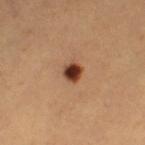Q: Is there a histopathology result?
A: catalogued during a skin exam; not biopsied
Q: Automated lesion metrics?
A: two-axis asymmetry of about 0.2; a mean CIELAB color near L≈40 a*≈24 b*≈32; a border-irregularity rating of about 1.5/10 and a color-variation rating of about 3.5/10; an automated nevus-likeness rating near 100 out of 100
Q: Patient demographics?
A: female, in their mid-50s
Q: What is the imaging modality?
A: ~15 mm crop, total-body skin-cancer survey
Q: What lighting was used for the tile?
A: cross-polarized
Q: What is the anatomic site?
A: the left thigh